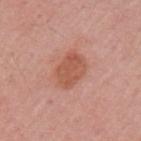Clinical summary: Cropped from a total-body skin-imaging series; the visible field is about 15 mm. A female subject aged 53 to 57. The lesion is on the left upper arm. The tile uses white-light illumination. Automated image analysis of the tile measured an area of roughly 10 mm², a shape eccentricity near 0.7, and a shape-asymmetry score of about 0.2 (0 = symmetric). It also reported a lesion color around L≈55 a*≈26 b*≈31 in CIELAB, about 9 CIELAB-L* units darker than the surrounding skin, and a normalized border contrast of about 7. Approximately 4 mm at its widest.